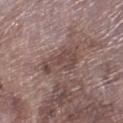notes: total-body-photography surveillance lesion; no biopsy | diameter: ~4.5 mm (longest diameter) | site: the right lower leg | image source: ~15 mm crop, total-body skin-cancer survey | tile lighting: white-light | patient: male, roughly 75 years of age.